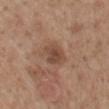size: about 3 mm
tile lighting: white-light
body site: the mid back
patient: male, approximately 60 years of age
imaging modality: 15 mm crop, total-body photography
TBP lesion metrics: a border-irregularity rating of about 2.5/10, internal color variation of about 3 on a 0–10 scale, and a peripheral color-asymmetry measure near 1; an automated nevus-likeness rating near 40 out of 100 and a lesion-detection confidence of about 100/100